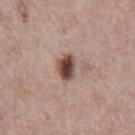<lesion>
<biopsy_status>not biopsied; imaged during a skin examination</biopsy_status>
<image>
  <source>total-body photography crop</source>
  <field_of_view_mm>15</field_of_view_mm>
</image>
<lighting>white-light</lighting>
<patient>
  <sex>male</sex>
  <age_approx>70</age_approx>
</patient>
<site>chest</site>
<automated_metrics>
  <cielab_L>46</cielab_L>
  <cielab_a>19</cielab_a>
  <cielab_b>23</cielab_b>
  <vs_skin_darker_L>17.0</vs_skin_darker_L>
  <border_irregularity_0_10>2.0</border_irregularity_0_10>
  <color_variation_0_10>7.0</color_variation_0_10>
  <peripheral_color_asymmetry>2.0</peripheral_color_asymmetry>
  <nevus_likeness_0_100>100</nevus_likeness_0_100>
  <lesion_detection_confidence_0_100>100</lesion_detection_confidence_0_100>
</automated_metrics>
<lesion_size>
  <long_diameter_mm_approx>3.5</long_diameter_mm_approx>
</lesion_size>
</lesion>